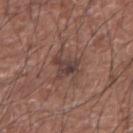No biopsy was performed on this lesion — it was imaged during a full skin examination and was not determined to be concerning. From the left upper arm. About 4 mm across. A male patient aged approximately 75. An algorithmic analysis of the crop reported an eccentricity of roughly 0.7 and a shape-asymmetry score of about 0.45 (0 = symmetric). It also reported a mean CIELAB color near L≈40 a*≈18 b*≈20, roughly 8 lightness units darker than nearby skin, and a lesion-to-skin contrast of about 7.5 (normalized; higher = more distinct). Cropped from a total-body skin-imaging series; the visible field is about 15 mm.